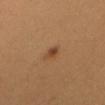patient: female, aged approximately 30
lesion size: ~2 mm (longest diameter)
site: the head or neck
automated metrics: a footprint of about 2 mm², a shape eccentricity near 0.75, and two-axis asymmetry of about 0.2; a mean CIELAB color near L≈45 a*≈22 b*≈35, about 10 CIELAB-L* units darker than the surrounding skin, and a normalized lesion–skin contrast near 8
image: 15 mm crop, total-body photography